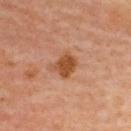lesion diameter = ~3 mm (longest diameter) | tile lighting = cross-polarized illumination | site = the upper back | acquisition = total-body-photography crop, ~15 mm field of view | patient = female, aged 58 to 62.